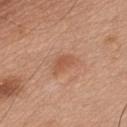| field | value |
|---|---|
| subject | male, aged 43–47 |
| body site | the chest |
| imaging modality | ~15 mm crop, total-body skin-cancer survey |
| lesion diameter | ≈3 mm |
| tile lighting | white-light |